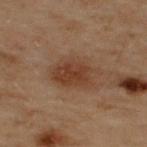workup: imaged on a skin check; not biopsied
anatomic site: the back
patient: male, aged approximately 50
image source: 15 mm crop, total-body photography